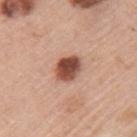No biopsy was performed on this lesion — it was imaged during a full skin examination and was not determined to be concerning.